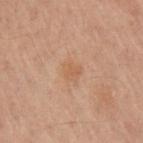biopsy status: imaged on a skin check; not biopsied
image: total-body-photography crop, ~15 mm field of view
automated lesion analysis: a lesion color around L≈43 a*≈16 b*≈26 in CIELAB, about 4 CIELAB-L* units darker than the surrounding skin, and a normalized border contrast of about 4.5; a border-irregularity rating of about 2.5/10, a within-lesion color-variation index near 1/10, and peripheral color asymmetry of about 0.5
patient: male, aged 58–62
body site: the front of the torso